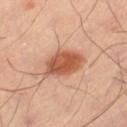The lesion was tiled from a total-body skin photograph and was not biopsied. This is a cross-polarized tile. On the left thigh. A 15 mm close-up tile from a total-body photography series done for melanoma screening.